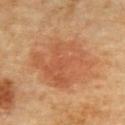follow-up: total-body-photography surveillance lesion; no biopsy | acquisition: total-body-photography crop, ~15 mm field of view | illumination: cross-polarized | automated metrics: a footprint of about 40 mm² and an eccentricity of roughly 0.7; a border-irregularity rating of about 3/10 and radial color variation of about 1.5 | patient: male, aged 83 or over | lesion diameter: ~9 mm (longest diameter) | location: the back.